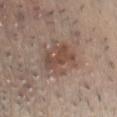On the chest.
A 15 mm close-up extracted from a 3D total-body photography capture.
A male subject, roughly 35 years of age.
Imaged with white-light lighting.